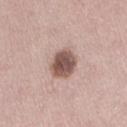No biopsy was performed on this lesion — it was imaged during a full skin examination and was not determined to be concerning.
The lesion is on the right thigh.
Cropped from a whole-body photographic skin survey; the tile spans about 15 mm.
The subject is a female about 50 years old.